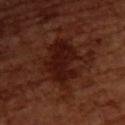The lesion was tiled from a total-body skin photograph and was not biopsied. About 6.5 mm across. Automated image analysis of the tile measured a mean CIELAB color near L≈20 a*≈23 b*≈24 and a lesion–skin lightness drop of about 7. The software also gave border irregularity of about 6.5 on a 0–10 scale, a within-lesion color-variation index near 3.5/10, and a peripheral color-asymmetry measure near 1. A close-up tile cropped from a whole-body skin photograph, about 15 mm across. The lesion is located on the upper back. A female patient, aged 53–57. Imaged with cross-polarized lighting.